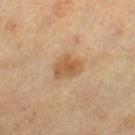Captured during whole-body skin photography for melanoma surveillance; the lesion was not biopsied.
Cropped from a whole-body photographic skin survey; the tile spans about 15 mm.
A female patient, about 40 years old.
Measured at roughly 3.5 mm in maximum diameter.
Imaged with cross-polarized lighting.
Located on the right lower leg.